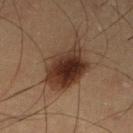Captured during whole-body skin photography for melanoma surveillance; the lesion was not biopsied. The patient is a male in their mid- to late 30s. Imaged with cross-polarized lighting. The lesion's longest dimension is about 7.5 mm. From the left lower leg. A roughly 15 mm field-of-view crop from a total-body skin photograph.